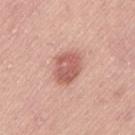The lesion was tiled from a total-body skin photograph and was not biopsied. On the left thigh. The subject is a female aged 38 to 42. Longest diameter approximately 4 mm. Automated tile analysis of the lesion measured an area of roughly 10 mm² and an outline eccentricity of about 0.65 (0 = round, 1 = elongated). The analysis additionally found a border-irregularity index near 2/10. The software also gave lesion-presence confidence of about 100/100. A close-up tile cropped from a whole-body skin photograph, about 15 mm across.